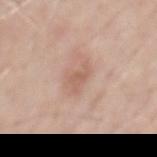<record>
  <biopsy_status>not biopsied; imaged during a skin examination</biopsy_status>
  <automated_metrics>
    <cielab_L>60</cielab_L>
    <cielab_a>20</cielab_a>
    <cielab_b>28</cielab_b>
    <vs_skin_contrast_norm>5.5</vs_skin_contrast_norm>
  </automated_metrics>
  <patient>
    <sex>male</sex>
    <age_approx>60</age_approx>
  </patient>
  <site>chest</site>
  <lesion_size>
    <long_diameter_mm_approx>3.0</long_diameter_mm_approx>
  </lesion_size>
  <image>
    <source>total-body photography crop</source>
    <field_of_view_mm>15</field_of_view_mm>
  </image>
</record>